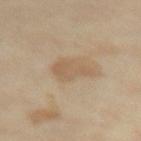biopsy status=no biopsy performed (imaged during a skin exam)
patient=female, roughly 50 years of age
site=the left thigh
lesion diameter=~4 mm (longest diameter)
automated metrics=a nevus-likeness score of about 0/100 and a detector confidence of about 100 out of 100 that the crop contains a lesion
acquisition=15 mm crop, total-body photography